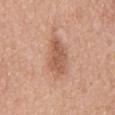follow-up: imaged on a skin check; not biopsied | image source: 15 mm crop, total-body photography | anatomic site: the mid back | lesion diameter: about 5.5 mm | illumination: white-light | subject: female, approximately 40 years of age.